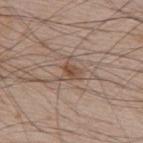This lesion was catalogued during total-body skin photography and was not selected for biopsy. About 2.5 mm across. The lesion-visualizer software estimated a lesion area of about 4 mm², an outline eccentricity of about 0.55 (0 = round, 1 = elongated), and a shape-asymmetry score of about 0.45 (0 = symmetric). The software also gave a lesion color around L≈49 a*≈17 b*≈27 in CIELAB and roughly 8 lightness units darker than nearby skin. And it measured a border-irregularity rating of about 5.5/10, a within-lesion color-variation index near 3.5/10, and a peripheral color-asymmetry measure near 1. The patient is a male aged approximately 65. The tile uses white-light illumination. This image is a 15 mm lesion crop taken from a total-body photograph. On the upper back.